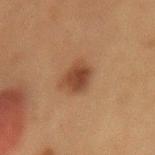biopsy_status: not biopsied; imaged during a skin examination
image:
  source: total-body photography crop
  field_of_view_mm: 15
site: lower back
lesion_size:
  long_diameter_mm_approx: 3.5
patient:
  sex: female
  age_approx: 50